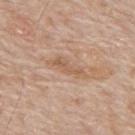Recorded during total-body skin imaging; not selected for excision or biopsy. Captured under white-light illumination. On the mid back. A 15 mm close-up tile from a total-body photography series done for melanoma screening. The lesion-visualizer software estimated a border-irregularity index near 5.5/10, a within-lesion color-variation index near 1.5/10, and peripheral color asymmetry of about 0.5. A male patient, roughly 65 years of age.